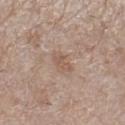This lesion was catalogued during total-body skin photography and was not selected for biopsy.
On the right lower leg.
A lesion tile, about 15 mm wide, cut from a 3D total-body photograph.
About 2.5 mm across.
A female patient in their mid-50s.
Captured under white-light illumination.
Automated image analysis of the tile measured a footprint of about 2.5 mm² and an outline eccentricity of about 0.85 (0 = round, 1 = elongated). The software also gave a color-variation rating of about 0/10 and peripheral color asymmetry of about 0.